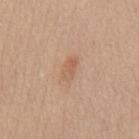This lesion was catalogued during total-body skin photography and was not selected for biopsy.